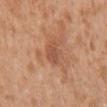workup — no biopsy performed (imaged during a skin exam); lesion diameter — about 3 mm; site — the right upper arm; image source — ~15 mm crop, total-body skin-cancer survey; patient — female, aged approximately 30.